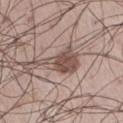Recorded during total-body skin imaging; not selected for excision or biopsy.
A male patient roughly 60 years of age.
A close-up tile cropped from a whole-body skin photograph, about 15 mm across.
Located on the leg.
Longest diameter approximately 5.5 mm.